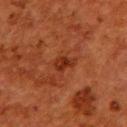follow-up=no biopsy performed (imaged during a skin exam).